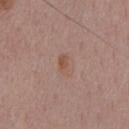The lesion was photographed on a routine skin check and not biopsied; there is no pathology result. This is a white-light tile. A region of skin cropped from a whole-body photographic capture, roughly 15 mm wide. A male patient, aged around 55. Measured at roughly 2.5 mm in maximum diameter. On the chest.